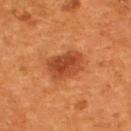Recorded during total-body skin imaging; not selected for excision or biopsy. Cropped from a whole-body photographic skin survey; the tile spans about 15 mm. Captured under cross-polarized illumination. Located on the upper back. The patient is a male roughly 55 years of age. Automated tile analysis of the lesion measured a lesion–skin lightness drop of about 11 and a normalized lesion–skin contrast near 8. The analysis additionally found a border-irregularity rating of about 2/10, internal color variation of about 3.5 on a 0–10 scale, and a peripheral color-asymmetry measure near 1. And it measured an automated nevus-likeness rating near 95 out of 100.